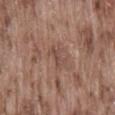The lesion was photographed on a routine skin check and not biopsied; there is no pathology result.
A male subject in their mid- to late 70s.
About 3 mm across.
A close-up tile cropped from a whole-body skin photograph, about 15 mm across.
The lesion is on the lower back.
Automated image analysis of the tile measured a border-irregularity index near 5/10, a within-lesion color-variation index near 0/10, and a peripheral color-asymmetry measure near 0. And it measured lesion-presence confidence of about 100/100.